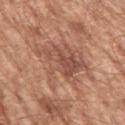Recorded during total-body skin imaging; not selected for excision or biopsy.
Longest diameter approximately 6 mm.
On the left upper arm.
A male patient, aged 63 to 67.
A close-up tile cropped from a whole-body skin photograph, about 15 mm across.